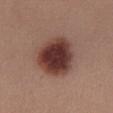The lesion was photographed on a routine skin check and not biopsied; there is no pathology result. Measured at roughly 5.5 mm in maximum diameter. A roughly 15 mm field-of-view crop from a total-body skin photograph. The patient is a female approximately 35 years of age. The tile uses white-light illumination. Automated image analysis of the tile measured a mean CIELAB color near L≈37 a*≈22 b*≈23, roughly 17 lightness units darker than nearby skin, and a normalized border contrast of about 13.5. And it measured a nevus-likeness score of about 100/100 and a detector confidence of about 100 out of 100 that the crop contains a lesion. The lesion is on the chest.